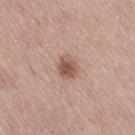Assessment: Part of a total-body skin-imaging series; this lesion was reviewed on a skin check and was not flagged for biopsy. Background: A roughly 15 mm field-of-view crop from a total-body skin photograph. About 2.5 mm across. This is a white-light tile. The lesion is located on the right thigh. A female patient aged 48 to 52. Automated tile analysis of the lesion measured a classifier nevus-likeness of about 95/100 and a lesion-detection confidence of about 100/100.